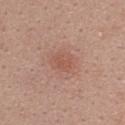Recorded during total-body skin imaging; not selected for excision or biopsy. Located on the upper back. The patient is a female aged 38–42. A 15 mm close-up extracted from a 3D total-body photography capture.